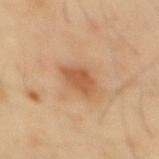Impression: Imaged during a routine full-body skin examination; the lesion was not biopsied and no histopathology is available. Background: A male patient, aged 38–42. Cropped from a whole-body photographic skin survey; the tile spans about 15 mm. Located on the mid back. Automated tile analysis of the lesion measured an area of roughly 7 mm², a shape eccentricity near 0.75, and two-axis asymmetry of about 0.2. And it measured a lesion color around L≈56 a*≈23 b*≈37 in CIELAB and roughly 10 lightness units darker than nearby skin. Imaged with cross-polarized lighting.